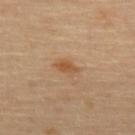Imaged during a routine full-body skin examination; the lesion was not biopsied and no histopathology is available. Measured at roughly 3 mm in maximum diameter. Automated tile analysis of the lesion measured a border-irregularity index near 3.5/10, a color-variation rating of about 0.5/10, and a peripheral color-asymmetry measure near 0. The software also gave an automated nevus-likeness rating near 75 out of 100 and a detector confidence of about 100 out of 100 that the crop contains a lesion. A female patient, in their mid-60s. Located on the upper back. A 15 mm close-up tile from a total-body photography series done for melanoma screening.